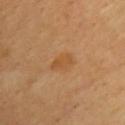Impression:
Part of a total-body skin-imaging series; this lesion was reviewed on a skin check and was not flagged for biopsy.
Image and clinical context:
A male subject aged 63 to 67. Automated tile analysis of the lesion measured an outline eccentricity of about 0.75 (0 = round, 1 = elongated) and a shape-asymmetry score of about 0.3 (0 = symmetric). It also reported about 6 CIELAB-L* units darker than the surrounding skin and a normalized border contrast of about 5.5. The analysis additionally found a border-irregularity rating of about 3/10, internal color variation of about 2 on a 0–10 scale, and a peripheral color-asymmetry measure near 0.5. The recorded lesion diameter is about 3 mm. This image is a 15 mm lesion crop taken from a total-body photograph. On the front of the torso.